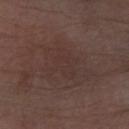Captured during whole-body skin photography for melanoma surveillance; the lesion was not biopsied.